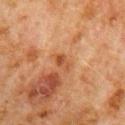<lesion>
  <biopsy_status>not biopsied; imaged during a skin examination</biopsy_status>
  <lighting>cross-polarized</lighting>
  <image>
    <source>total-body photography crop</source>
    <field_of_view_mm>15</field_of_view_mm>
  </image>
  <site>right upper arm</site>
  <lesion_size>
    <long_diameter_mm_approx>2.5</long_diameter_mm_approx>
  </lesion_size>
  <automated_metrics>
    <vs_skin_darker_L>7.0</vs_skin_darker_L>
    <vs_skin_contrast_norm>6.5</vs_skin_contrast_norm>
    <border_irregularity_0_10>2.5</border_irregularity_0_10>
    <color_variation_0_10>2.5</color_variation_0_10>
    <peripheral_color_asymmetry>0.5</peripheral_color_asymmetry>
    <nevus_likeness_0_100>0</nevus_likeness_0_100>
    <lesion_detection_confidence_0_100>100</lesion_detection_confidence_0_100>
  </automated_metrics>
  <patient>
    <sex>male</sex>
    <age_approx>80</age_approx>
  </patient>
</lesion>